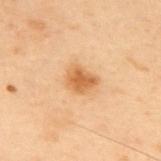This lesion was catalogued during total-body skin photography and was not selected for biopsy. From the back. This is a cross-polarized tile. A male patient aged around 55. Cropped from a total-body skin-imaging series; the visible field is about 15 mm. Longest diameter approximately 3 mm.